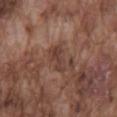notes = imaged on a skin check; not biopsied | acquisition = ~15 mm crop, total-body skin-cancer survey | patient = male, aged 73 to 77 | automated metrics = an area of roughly 5.5 mm² and two-axis asymmetry of about 0.25; border irregularity of about 3.5 on a 0–10 scale and a peripheral color-asymmetry measure near 1; a classifier nevus-likeness of about 0/100 and lesion-presence confidence of about 95/100 | location = the mid back | diameter = ≈3.5 mm.